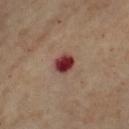Q: Is there a histopathology result?
A: total-body-photography surveillance lesion; no biopsy
Q: Automated lesion metrics?
A: an area of roughly 5 mm², an eccentricity of roughly 0.4, and a symmetry-axis asymmetry near 0.15; a lesion color around L≈37 a*≈30 b*≈23 in CIELAB, roughly 18 lightness units darker than nearby skin, and a lesion-to-skin contrast of about 14 (normalized; higher = more distinct); an automated nevus-likeness rating near 0 out of 100 and a detector confidence of about 100 out of 100 that the crop contains a lesion
Q: What is the anatomic site?
A: the left forearm
Q: What are the patient's age and sex?
A: female, roughly 55 years of age
Q: How was the tile lit?
A: cross-polarized
Q: Lesion size?
A: ≈2.5 mm
Q: How was this image acquired?
A: 15 mm crop, total-body photography